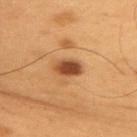Q: Was a biopsy performed?
A: imaged on a skin check; not biopsied
Q: Lesion location?
A: the upper back
Q: What lighting was used for the tile?
A: cross-polarized
Q: What is the imaging modality?
A: ~15 mm tile from a whole-body skin photo
Q: Who is the patient?
A: male, in their mid- to late 50s
Q: Lesion size?
A: ≈3.5 mm
Q: Automated lesion metrics?
A: a footprint of about 5.5 mm² and a shape eccentricity near 0.8; roughly 16 lightness units darker than nearby skin and a normalized lesion–skin contrast near 11